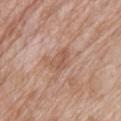workup=total-body-photography surveillance lesion; no biopsy | lighting=white-light illumination | image source=15 mm crop, total-body photography | anatomic site=the upper back | subject=female, aged 68–72.